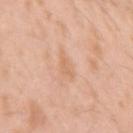* notes — imaged on a skin check; not biopsied
* lesion size — ~3 mm (longest diameter)
* illumination — white-light illumination
* site — the left upper arm
* patient — male, roughly 25 years of age
* image — 15 mm crop, total-body photography